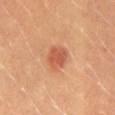The lesion was photographed on a routine skin check and not biopsied; there is no pathology result. Imaged with cross-polarized lighting. A female subject in their 30s. Automated tile analysis of the lesion measured a lesion area of about 7 mm², an eccentricity of roughly 0.6, and a symmetry-axis asymmetry near 0.15. It also reported a nevus-likeness score of about 95/100 and a detector confidence of about 100 out of 100 that the crop contains a lesion. On the lower back. Measured at roughly 3.5 mm in maximum diameter. A 15 mm crop from a total-body photograph taken for skin-cancer surveillance.